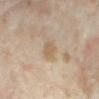Recorded during total-body skin imaging; not selected for excision or biopsy. The lesion is located on the right forearm. The subject is a female approximately 35 years of age. A 15 mm close-up extracted from a 3D total-body photography capture.